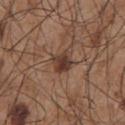Imaged during a routine full-body skin examination; the lesion was not biopsied and no histopathology is available. A male subject, aged 53 to 57. A 15 mm close-up extracted from a 3D total-body photography capture. The lesion is located on the chest.